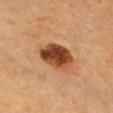This lesion was catalogued during total-body skin photography and was not selected for biopsy.
A subject in their 70s.
The recorded lesion diameter is about 5 mm.
Located on the front of the torso.
The tile uses cross-polarized illumination.
A roughly 15 mm field-of-view crop from a total-body skin photograph.